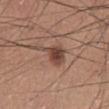  biopsy_status: not biopsied; imaged during a skin examination
  site: abdomen
  patient:
    sex: male
    age_approx: 25
  automated_metrics:
    vs_skin_contrast_norm: 9.0
    border_irregularity_0_10: 5.0
  image:
    source: total-body photography crop
    field_of_view_mm: 15
  lighting: white-light